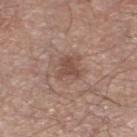<lesion>
  <biopsy_status>not biopsied; imaged during a skin examination</biopsy_status>
  <image>
    <source>total-body photography crop</source>
    <field_of_view_mm>15</field_of_view_mm>
  </image>
  <patient>
    <sex>male</sex>
    <age_approx>65</age_approx>
  </patient>
  <lighting>white-light</lighting>
  <lesion_size>
    <long_diameter_mm_approx>3.0</long_diameter_mm_approx>
  </lesion_size>
  <site>left lower leg</site>
</lesion>